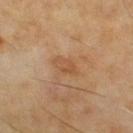Recorded during total-body skin imaging; not selected for excision or biopsy. Measured at roughly 3.5 mm in maximum diameter. An algorithmic analysis of the crop reported border irregularity of about 3 on a 0–10 scale. And it measured an automated nevus-likeness rating near 0 out of 100 and lesion-presence confidence of about 100/100. A male patient about 55 years old. Cropped from a total-body skin-imaging series; the visible field is about 15 mm. From the upper back. This is a cross-polarized tile.